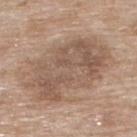Findings:
– notes: imaged on a skin check; not biopsied
– TBP lesion metrics: a footprint of about 40 mm², an outline eccentricity of about 0.8 (0 = round, 1 = elongated), and a shape-asymmetry score of about 0.2 (0 = symmetric); a lesion color around L≈54 a*≈16 b*≈27 in CIELAB, a lesion–skin lightness drop of about 10, and a normalized lesion–skin contrast near 6.5; an automated nevus-likeness rating near 5 out of 100 and a lesion-detection confidence of about 100/100
– tile lighting: white-light illumination
– anatomic site: the back
– subject: female, approximately 75 years of age
– diameter: ≈10 mm
– acquisition: ~15 mm crop, total-body skin-cancer survey